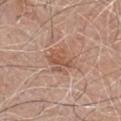follow-up = imaged on a skin check; not biopsied | tile lighting = white-light | site = the chest | size = ≈3 mm | imaging modality = ~15 mm tile from a whole-body skin photo | subject = male, aged approximately 80.